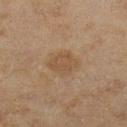Q: Was a biopsy performed?
A: total-body-photography surveillance lesion; no biopsy
Q: Illumination type?
A: cross-polarized illumination
Q: Who is the patient?
A: female, aged around 60
Q: What kind of image is this?
A: 15 mm crop, total-body photography
Q: What is the anatomic site?
A: the left lower leg